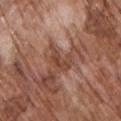Measured at roughly 4 mm in maximum diameter. The total-body-photography lesion software estimated a footprint of about 6 mm², an outline eccentricity of about 0.8 (0 = round, 1 = elongated), and a symmetry-axis asymmetry near 0.5. The analysis additionally found a mean CIELAB color near L≈45 a*≈22 b*≈28 and a normalized lesion–skin contrast near 6. It also reported a classifier nevus-likeness of about 0/100 and lesion-presence confidence of about 80/100. A region of skin cropped from a whole-body photographic capture, roughly 15 mm wide. The patient is a male approximately 70 years of age. The lesion is on the chest.